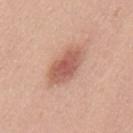Q: Was this lesion biopsied?
A: total-body-photography surveillance lesion; no biopsy
Q: What is the anatomic site?
A: the back
Q: What kind of image is this?
A: 15 mm crop, total-body photography
Q: Patient demographics?
A: female, approximately 40 years of age
Q: Lesion size?
A: ~5.5 mm (longest diameter)
Q: What lighting was used for the tile?
A: white-light